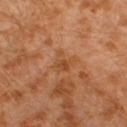{"biopsy_status": "not biopsied; imaged during a skin examination", "image": {"source": "total-body photography crop", "field_of_view_mm": 15}, "automated_metrics": {"cielab_L": 47, "cielab_a": 24, "cielab_b": 37, "vs_skin_contrast_norm": 6.0, "border_irregularity_0_10": 4.5}, "patient": {"sex": "male", "age_approx": 30}, "lesion_size": {"long_diameter_mm_approx": 3.0}, "lighting": "cross-polarized", "site": "left lower leg"}